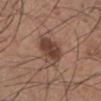follow-up = total-body-photography surveillance lesion; no biopsy | anatomic site = the left lower leg | image source = ~15 mm tile from a whole-body skin photo | patient = male, aged approximately 35.